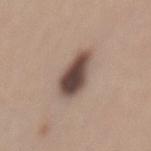The total-body-photography lesion software estimated an area of roughly 11 mm², an eccentricity of roughly 0.65, and a symmetry-axis asymmetry near 0.25. The analysis additionally found an average lesion color of about L≈46 a*≈15 b*≈21 (CIELAB), a lesion–skin lightness drop of about 18, and a normalized border contrast of about 12.5. It also reported a classifier nevus-likeness of about 80/100 and a lesion-detection confidence of about 100/100. The lesion is on the mid back. A male patient in their mid-50s. Longest diameter approximately 4 mm. This is a white-light tile. Cropped from a total-body skin-imaging series; the visible field is about 15 mm.